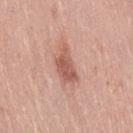Part of a total-body skin-imaging series; this lesion was reviewed on a skin check and was not flagged for biopsy. Located on the lower back. A female patient approximately 40 years of age. A lesion tile, about 15 mm wide, cut from a 3D total-body photograph. An algorithmic analysis of the crop reported a lesion area of about 7.5 mm², a shape eccentricity near 0.85, and a symmetry-axis asymmetry near 0.3. And it measured a border-irregularity rating of about 3.5/10, a within-lesion color-variation index near 2.5/10, and peripheral color asymmetry of about 1. Measured at roughly 4.5 mm in maximum diameter. This is a white-light tile.